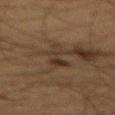Impression: Captured during whole-body skin photography for melanoma surveillance; the lesion was not biopsied. Image and clinical context: Captured under cross-polarized illumination. On the mid back. The patient is a male aged 63 to 67. A region of skin cropped from a whole-body photographic capture, roughly 15 mm wide.